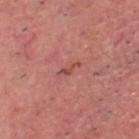Assessment:
Imaged during a routine full-body skin examination; the lesion was not biopsied and no histopathology is available.
Image and clinical context:
A male patient, aged around 70. From the head or neck. Automated tile analysis of the lesion measured an average lesion color of about L≈49 a*≈29 b*≈27 (CIELAB) and a lesion–skin lightness drop of about 8. It also reported a lesion-detection confidence of about 95/100. The tile uses white-light illumination. A 15 mm close-up tile from a total-body photography series done for melanoma screening.